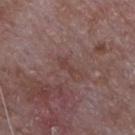The lesion was photographed on a routine skin check and not biopsied; there is no pathology result. A male patient about 65 years old. Cropped from a whole-body photographic skin survey; the tile spans about 15 mm. Located on the front of the torso.